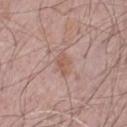The lesion was tiled from a total-body skin photograph and was not biopsied.
A roughly 15 mm field-of-view crop from a total-body skin photograph.
The lesion is located on the chest.
Automated image analysis of the tile measured a lesion color around L≈56 a*≈20 b*≈26 in CIELAB and a normalized border contrast of about 6. The analysis additionally found a border-irregularity index near 2.5/10 and a peripheral color-asymmetry measure near 0.5.
A male patient, roughly 65 years of age.
Measured at roughly 3 mm in maximum diameter.
Captured under white-light illumination.